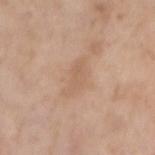Clinical impression: The lesion was photographed on a routine skin check and not biopsied; there is no pathology result. Image and clinical context: A male subject, aged 58–62. Imaged with white-light lighting. From the right upper arm. A 15 mm close-up tile from a total-body photography series done for melanoma screening. The lesion-visualizer software estimated a lesion area of about 6 mm², an outline eccentricity of about 0.9 (0 = round, 1 = elongated), and a symmetry-axis asymmetry near 0.4. And it measured a mean CIELAB color near L≈60 a*≈18 b*≈31, a lesion–skin lightness drop of about 7, and a lesion-to-skin contrast of about 4.5 (normalized; higher = more distinct). The software also gave internal color variation of about 1.5 on a 0–10 scale. It also reported a nevus-likeness score of about 0/100 and a lesion-detection confidence of about 100/100.